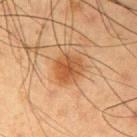lesion diameter=≈3.5 mm; acquisition=total-body-photography crop, ~15 mm field of view; subject=male, aged 53–57; anatomic site=the left upper arm.